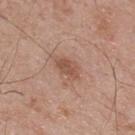Imaged during a routine full-body skin examination; the lesion was not biopsied and no histopathology is available. The lesion's longest dimension is about 3 mm. Imaged with white-light lighting. On the upper back. A male patient roughly 70 years of age. A lesion tile, about 15 mm wide, cut from a 3D total-body photograph. An algorithmic analysis of the crop reported a classifier nevus-likeness of about 45/100 and lesion-presence confidence of about 100/100.